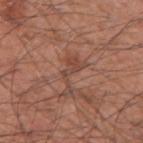{"biopsy_status": "not biopsied; imaged during a skin examination", "site": "right upper arm", "lesion_size": {"long_diameter_mm_approx": 5.0}, "image": {"source": "total-body photography crop", "field_of_view_mm": 15}, "patient": {"sex": "male", "age_approx": 60}, "automated_metrics": {"cielab_L": 45, "cielab_a": 21, "cielab_b": 27, "vs_skin_darker_L": 7.0, "vs_skin_contrast_norm": 5.5, "border_irregularity_0_10": 10.0, "peripheral_color_asymmetry": 0.5, "lesion_detection_confidence_0_100": 95}, "lighting": "white-light"}